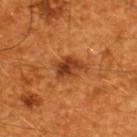| feature | finding |
|---|---|
| biopsy status | catalogued during a skin exam; not biopsied |
| acquisition | 15 mm crop, total-body photography |
| size | ≈4 mm |
| anatomic site | the upper back |
| subject | male, aged approximately 60 |
| lighting | cross-polarized illumination |
| automated metrics | a lesion area of about 8.5 mm² and a shape-asymmetry score of about 0.25 (0 = symmetric); a mean CIELAB color near L≈39 a*≈26 b*≈38, roughly 10 lightness units darker than nearby skin, and a lesion-to-skin contrast of about 8 (normalized; higher = more distinct); border irregularity of about 3 on a 0–10 scale, internal color variation of about 5.5 on a 0–10 scale, and radial color variation of about 2; a classifier nevus-likeness of about 75/100 and a lesion-detection confidence of about 100/100 |